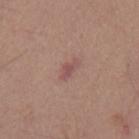{"lighting": "white-light", "lesion_size": {"long_diameter_mm_approx": 3.0}, "image": {"source": "total-body photography crop", "field_of_view_mm": 15}, "site": "back", "patient": {"sex": "male", "age_approx": 55}}